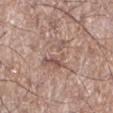<lesion>
<biopsy_status>not biopsied; imaged during a skin examination</biopsy_status>
<patient>
  <sex>male</sex>
  <age_approx>60</age_approx>
</patient>
<site>left lower leg</site>
<image>
  <source>total-body photography crop</source>
  <field_of_view_mm>15</field_of_view_mm>
</image>
<lighting>white-light</lighting>
<lesion_size>
  <long_diameter_mm_approx>6.0</long_diameter_mm_approx>
</lesion_size>
</lesion>